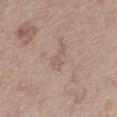workup = catalogued during a skin exam; not biopsied | subject = female, approximately 70 years of age | diameter = ~3 mm (longest diameter) | tile lighting = white-light | imaging modality = 15 mm crop, total-body photography | location = the left lower leg.